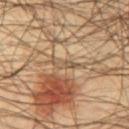No biopsy was performed on this lesion — it was imaged during a full skin examination and was not determined to be concerning. A region of skin cropped from a whole-body photographic capture, roughly 15 mm wide. Measured at roughly 3.5 mm in maximum diameter. The subject is a male aged 58 to 62. This is a cross-polarized tile. From the chest.